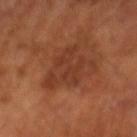This lesion was catalogued during total-body skin photography and was not selected for biopsy. A roughly 15 mm field-of-view crop from a total-body skin photograph. A male patient, aged around 65.